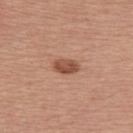Clinical impression:
No biopsy was performed on this lesion — it was imaged during a full skin examination and was not determined to be concerning.
Background:
From the back. This is a white-light tile. A female subject, in their mid-60s. A roughly 15 mm field-of-view crop from a total-body skin photograph.